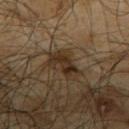An algorithmic analysis of the crop reported an outline eccentricity of about 0.85 (0 = round, 1 = elongated) and two-axis asymmetry of about 0.4. The analysis additionally found a border-irregularity index near 4.5/10 and a within-lesion color-variation index near 3.5/10. The software also gave lesion-presence confidence of about 80/100.
The lesion is located on the upper back.
Captured under cross-polarized illumination.
The subject is a male aged 63–67.
A roughly 15 mm field-of-view crop from a total-body skin photograph.